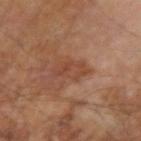biopsy status = total-body-photography surveillance lesion; no biopsy
subject = male, roughly 65 years of age
location = the left arm
imaging modality = ~15 mm crop, total-body skin-cancer survey